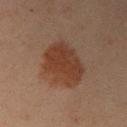notes: imaged on a skin check; not biopsied | automated metrics: an area of roughly 20 mm², an eccentricity of roughly 0.5, and two-axis asymmetry of about 0.15; a border-irregularity rating of about 2/10, a color-variation rating of about 3/10, and peripheral color asymmetry of about 1; a nevus-likeness score of about 100/100 | illumination: cross-polarized illumination | location: the right upper arm | size: ~5.5 mm (longest diameter) | acquisition: ~15 mm crop, total-body skin-cancer survey | subject: male, approximately 60 years of age.